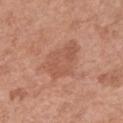| field | value |
|---|---|
| workup | catalogued during a skin exam; not biopsied |
| patient | female, in their 50s |
| lesion size | ~5.5 mm (longest diameter) |
| image source | ~15 mm crop, total-body skin-cancer survey |
| location | the front of the torso |